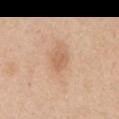Impression: The lesion was tiled from a total-body skin photograph and was not biopsied. Acquisition and patient details: A region of skin cropped from a whole-body photographic capture, roughly 15 mm wide. The lesion is on the mid back. The tile uses white-light illumination. The patient is a male approximately 60 years of age.